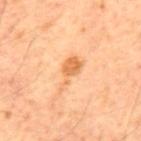Part of a total-body skin-imaging series; this lesion was reviewed on a skin check and was not flagged for biopsy.
A lesion tile, about 15 mm wide, cut from a 3D total-body photograph.
A male subject aged 58–62.
On the back.
The lesion's longest dimension is about 4.5 mm.